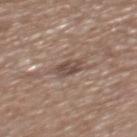- follow-up: imaged on a skin check; not biopsied
- subject: male, in their mid- to late 60s
- automated lesion analysis: an eccentricity of roughly 0.65 and a shape-asymmetry score of about 0.25 (0 = symmetric); a lesion color around L≈47 a*≈15 b*≈23 in CIELAB, a lesion–skin lightness drop of about 10, and a normalized lesion–skin contrast near 7.5; border irregularity of about 2 on a 0–10 scale, a within-lesion color-variation index near 3/10, and radial color variation of about 1
- location: the back
- lesion size: ≈3 mm
- lighting: white-light
- acquisition: ~15 mm tile from a whole-body skin photo